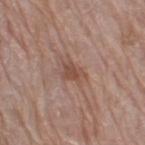<lesion>
<biopsy_status>not biopsied; imaged during a skin examination</biopsy_status>
<lighting>white-light</lighting>
<patient>
  <sex>female</sex>
  <age_approx>75</age_approx>
</patient>
<site>right thigh</site>
<image>
  <source>total-body photography crop</source>
  <field_of_view_mm>15</field_of_view_mm>
</image>
</lesion>